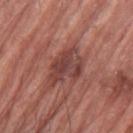Findings:
– image · ~15 mm crop, total-body skin-cancer survey
– illumination · white-light
– anatomic site · the left forearm
– subject · male, aged 68–72